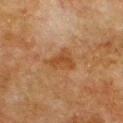Q: What lighting was used for the tile?
A: cross-polarized illumination
Q: What are the patient's age and sex?
A: male, roughly 80 years of age
Q: Lesion location?
A: the chest
Q: What is the lesion's diameter?
A: ≈4 mm
Q: What is the imaging modality?
A: ~15 mm crop, total-body skin-cancer survey